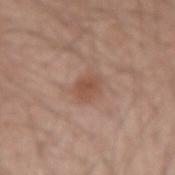Case summary:
* follow-up — total-body-photography surveillance lesion; no biopsy
* anatomic site — the right forearm
* size — about 2.5 mm
* subject — male, aged 53–57
* illumination — white-light illumination
* imaging modality — ~15 mm crop, total-body skin-cancer survey